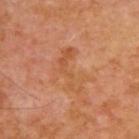  biopsy_status: not biopsied; imaged during a skin examination
  patient:
    sex: male
    age_approx: 55
  image:
    source: total-body photography crop
    field_of_view_mm: 15
  site: upper back
  automated_metrics:
    area_mm2_approx: 7.5
    eccentricity: 0.9
    vs_skin_darker_L: 6.0
    border_irregularity_0_10: 9.5
    color_variation_0_10: 4.0
    peripheral_color_asymmetry: 1.0
  lighting: cross-polarized
  lesion_size:
    long_diameter_mm_approx: 5.5